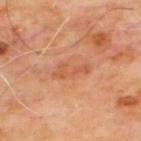Impression:
Captured during whole-body skin photography for melanoma surveillance; the lesion was not biopsied.
Acquisition and patient details:
Automated tile analysis of the lesion measured a mean CIELAB color near L≈54 a*≈27 b*≈37 and roughly 7 lightness units darker than nearby skin. The software also gave a lesion-detection confidence of about 100/100. Imaged with cross-polarized lighting. On the upper back. A close-up tile cropped from a whole-body skin photograph, about 15 mm across. A male subject about 65 years old.